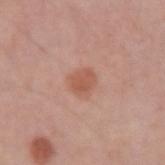Part of a total-body skin-imaging series; this lesion was reviewed on a skin check and was not flagged for biopsy. Measured at roughly 2.5 mm in maximum diameter. Captured under white-light illumination. This image is a 15 mm lesion crop taken from a total-body photograph. A male patient aged approximately 60. Automated tile analysis of the lesion measured a lesion color around L≈56 a*≈24 b*≈30 in CIELAB, a lesion–skin lightness drop of about 8, and a normalized border contrast of about 6.5. On the left upper arm.